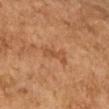Recorded during total-body skin imaging; not selected for excision or biopsy. About 3.5 mm across. The subject is a female in their 60s. A roughly 15 mm field-of-view crop from a total-body skin photograph. This is a cross-polarized tile. The lesion is on the left upper arm.